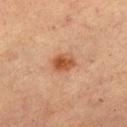<record>
  <biopsy_status>not biopsied; imaged during a skin examination</biopsy_status>
  <site>front of the torso</site>
  <image>
    <source>total-body photography crop</source>
    <field_of_view_mm>15</field_of_view_mm>
  </image>
  <patient>
    <sex>female</sex>
    <age_approx>60</age_approx>
  </patient>
</record>